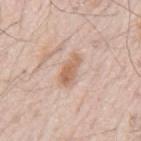This lesion was catalogued during total-body skin photography and was not selected for biopsy. The subject is a male aged 78 to 82. Measured at roughly 3.5 mm in maximum diameter. A 15 mm close-up tile from a total-body photography series done for melanoma screening. On the mid back. Captured under white-light illumination. The lesion-visualizer software estimated a border-irregularity rating of about 3/10, a within-lesion color-variation index near 3/10, and a peripheral color-asymmetry measure near 1. The software also gave a nevus-likeness score of about 10/100 and a detector confidence of about 100 out of 100 that the crop contains a lesion.